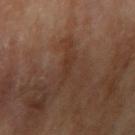This lesion was catalogued during total-body skin photography and was not selected for biopsy. A male subject approximately 85 years of age or older. The lesion is on the right upper arm. A 15 mm crop from a total-body photograph taken for skin-cancer surveillance.